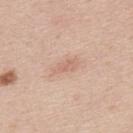– notes: catalogued during a skin exam; not biopsied
– automated metrics: a mean CIELAB color near L≈65 a*≈20 b*≈28; a detector confidence of about 100 out of 100 that the crop contains a lesion
– subject: male, in their mid- to late 40s
– body site: the back
– tile lighting: white-light
– imaging modality: 15 mm crop, total-body photography
– lesion diameter: about 3 mm